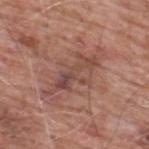Recorded during total-body skin imaging; not selected for excision or biopsy. The subject is a male in their 60s. About 6.5 mm across. This is a white-light tile. The lesion is located on the upper back. Cropped from a total-body skin-imaging series; the visible field is about 15 mm.